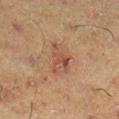| field | value |
|---|---|
| notes | catalogued during a skin exam; not biopsied |
| subject | male, in their 60s |
| lesion diameter | ≈4.5 mm |
| site | the right lower leg |
| automated metrics | an average lesion color of about L≈39 a*≈18 b*≈24 (CIELAB) and a lesion–skin lightness drop of about 7 |
| image | 15 mm crop, total-body photography |